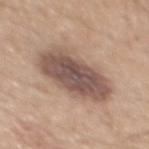Q: Was this lesion biopsied?
A: imaged on a skin check; not biopsied
Q: How was this image acquired?
A: total-body-photography crop, ~15 mm field of view
Q: Illumination type?
A: white-light illumination
Q: Lesion size?
A: ~7 mm (longest diameter)
Q: Who is the patient?
A: male, about 45 years old
Q: Where on the body is the lesion?
A: the back